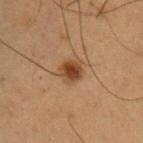  biopsy_status: not biopsied; imaged during a skin examination
  site: chest
  patient:
    sex: male
    age_approx: 50
  automated_metrics:
    area_mm2_approx: 5.0
    eccentricity: 0.4
    shape_asymmetry: 0.2
    cielab_L: 34
    cielab_a: 18
    cielab_b: 28
    vs_skin_darker_L: 11.0
    border_irregularity_0_10: 1.5
    color_variation_0_10: 3.0
    peripheral_color_asymmetry: 1.0
  lesion_size:
    long_diameter_mm_approx: 2.5
  image:
    source: total-body photography crop
    field_of_view_mm: 15
  lighting: cross-polarized